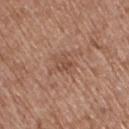notes = total-body-photography surveillance lesion; no biopsy | patient = female, about 75 years old | illumination = white-light | acquisition = ~15 mm tile from a whole-body skin photo | lesion diameter = ≈2.5 mm | location = the right thigh.